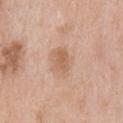Context:
An algorithmic analysis of the crop reported a lesion area of about 8.5 mm² and an outline eccentricity of about 0.6 (0 = round, 1 = elongated). And it measured border irregularity of about 2.5 on a 0–10 scale, a within-lesion color-variation index near 3/10, and radial color variation of about 1. And it measured a lesion-detection confidence of about 100/100. A 15 mm close-up extracted from a 3D total-body photography capture. The lesion is located on the upper back. The tile uses white-light illumination. The recorded lesion diameter is about 4 mm. The patient is a male aged approximately 40.